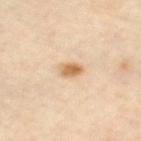Imaged during a routine full-body skin examination; the lesion was not biopsied and no histopathology is available. A female subject in their mid- to late 60s. About 2.5 mm across. Captured under cross-polarized illumination. The lesion is on the right thigh. This image is a 15 mm lesion crop taken from a total-body photograph.